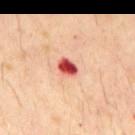workup = total-body-photography surveillance lesion; no biopsy | illumination = cross-polarized illumination | body site = the mid back | image source = 15 mm crop, total-body photography | size = about 2.5 mm | patient = male, aged approximately 30 | TBP lesion metrics = a lesion color around L≈54 a*≈38 b*≈32 in CIELAB and a normalized border contrast of about 13; a nevus-likeness score of about 0/100 and a detector confidence of about 100 out of 100 that the crop contains a lesion.